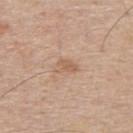Impression: This lesion was catalogued during total-body skin photography and was not selected for biopsy. Acquisition and patient details: The lesion's longest dimension is about 2.5 mm. The patient is a male aged 53 to 57. A close-up tile cropped from a whole-body skin photograph, about 15 mm across. Captured under white-light illumination. On the upper back.